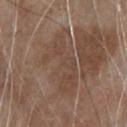Findings:
* workup — imaged on a skin check; not biopsied
* automated lesion analysis — a border-irregularity rating of about 6/10, internal color variation of about 3 on a 0–10 scale, and a peripheral color-asymmetry measure near 1; an automated nevus-likeness rating near 0 out of 100 and a detector confidence of about 75 out of 100 that the crop contains a lesion
* site — the chest
* subject — male, about 80 years old
* lighting — white-light illumination
* acquisition — ~15 mm crop, total-body skin-cancer survey